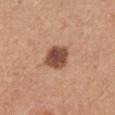biopsy status: no biopsy performed (imaged during a skin exam) | site: the right upper arm | subject: male, aged 28–32 | lesion diameter: about 3.5 mm | illumination: white-light illumination | image: ~15 mm crop, total-body skin-cancer survey | image-analysis metrics: an outline eccentricity of about 0.5 (0 = round, 1 = elongated) and two-axis asymmetry of about 0.2; an average lesion color of about L≈49 a*≈22 b*≈29 (CIELAB), roughly 16 lightness units darker than nearby skin, and a normalized border contrast of about 11; lesion-presence confidence of about 100/100.